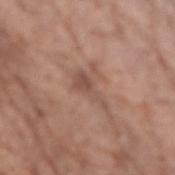This lesion was catalogued during total-body skin photography and was not selected for biopsy.
The lesion is located on the left forearm.
The subject is a male aged 68 to 72.
The recorded lesion diameter is about 4.5 mm.
This is a white-light tile.
Cropped from a whole-body photographic skin survey; the tile spans about 15 mm.